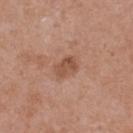Q: Is there a histopathology result?
A: imaged on a skin check; not biopsied
Q: Who is the patient?
A: female, aged approximately 40
Q: Lesion location?
A: the upper back
Q: How was this image acquired?
A: ~15 mm crop, total-body skin-cancer survey